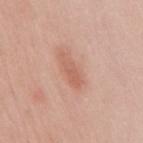Q: Was this lesion biopsied?
A: total-body-photography surveillance lesion; no biopsy
Q: What is the lesion's diameter?
A: ≈4 mm
Q: How was the tile lit?
A: white-light illumination
Q: Patient demographics?
A: female, aged 38–42
Q: Automated lesion metrics?
A: a mean CIELAB color near L≈60 a*≈24 b*≈30; an automated nevus-likeness rating near 80 out of 100 and a detector confidence of about 100 out of 100 that the crop contains a lesion
Q: What is the imaging modality?
A: 15 mm crop, total-body photography
Q: Where on the body is the lesion?
A: the mid back